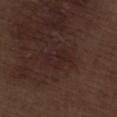No biopsy was performed on this lesion — it was imaged during a full skin examination and was not determined to be concerning.
The patient is a male about 70 years old.
The tile uses white-light illumination.
A 15 mm crop from a total-body photograph taken for skin-cancer surveillance.
An algorithmic analysis of the crop reported a footprint of about 6 mm², a shape eccentricity near 0.85, and a shape-asymmetry score of about 0.4 (0 = symmetric). And it measured a border-irregularity index near 4/10, internal color variation of about 3 on a 0–10 scale, and peripheral color asymmetry of about 1. And it measured an automated nevus-likeness rating near 5 out of 100.
The lesion is on the right thigh.
About 3.5 mm across.